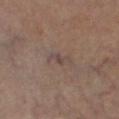<record>
<biopsy_status>not biopsied; imaged during a skin examination</biopsy_status>
<image>
  <source>total-body photography crop</source>
  <field_of_view_mm>15</field_of_view_mm>
</image>
<automated_metrics>
  <area_mm2_approx>3.5</area_mm2_approx>
  <eccentricity>0.9</eccentricity>
  <shape_asymmetry>0.5</shape_asymmetry>
  <lesion_detection_confidence_0_100>80</lesion_detection_confidence_0_100>
</automated_metrics>
<patient>
  <sex>male</sex>
  <age_approx>60</age_approx>
</patient>
<lesion_size>
  <long_diameter_mm_approx>3.0</long_diameter_mm_approx>
</lesion_size>
<lighting>cross-polarized</lighting>
<site>right lower leg</site>
</record>